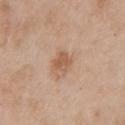Assessment: The lesion was tiled from a total-body skin photograph and was not biopsied. Clinical summary: Captured under white-light illumination. The total-body-photography lesion software estimated a footprint of about 4.5 mm², a shape eccentricity near 0.6, and a symmetry-axis asymmetry near 0.3. The software also gave border irregularity of about 3 on a 0–10 scale, a within-lesion color-variation index near 2.5/10, and a peripheral color-asymmetry measure near 1. A male subject, aged 58–62. On the chest. The recorded lesion diameter is about 2.5 mm. A 15 mm close-up extracted from a 3D total-body photography capture.